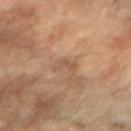Acquisition and patient details:
A female patient aged 68 to 72. The lesion is located on the right forearm. The tile uses cross-polarized illumination. A lesion tile, about 15 mm wide, cut from a 3D total-body photograph.